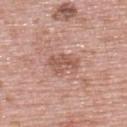follow-up = imaged on a skin check; not biopsied | illumination = white-light | imaging modality = 15 mm crop, total-body photography | diameter = ~4 mm (longest diameter) | anatomic site = the upper back | patient = female, aged 58 to 62.